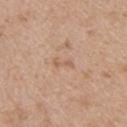The lesion was tiled from a total-body skin photograph and was not biopsied.
Located on the upper back.
A female patient about 45 years old.
A close-up tile cropped from a whole-body skin photograph, about 15 mm across.
Imaged with white-light lighting.